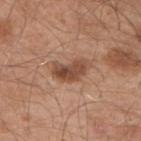The lesion was photographed on a routine skin check and not biopsied; there is no pathology result. A male subject, aged approximately 55. The lesion is located on the left upper arm. Imaged with white-light lighting. Approximately 4.5 mm at its widest. A lesion tile, about 15 mm wide, cut from a 3D total-body photograph.